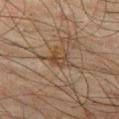<record>
  <biopsy_status>not biopsied; imaged during a skin examination</biopsy_status>
  <patient>
    <sex>male</sex>
    <age_approx>65</age_approx>
  </patient>
  <image>
    <source>total-body photography crop</source>
    <field_of_view_mm>15</field_of_view_mm>
  </image>
  <site>left thigh</site>
</record>